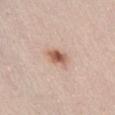Notes:
• workup: catalogued during a skin exam; not biopsied
• automated lesion analysis: a lesion color around L≈59 a*≈21 b*≈30 in CIELAB, roughly 13 lightness units darker than nearby skin, and a lesion-to-skin contrast of about 9 (normalized; higher = more distinct); lesion-presence confidence of about 100/100
• image source: 15 mm crop, total-body photography
• lighting: white-light illumination
• lesion size: ~3 mm (longest diameter)
• body site: the abdomen
• patient: male, aged 23–27